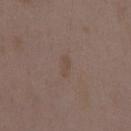workup — no biopsy performed (imaged during a skin exam) | image-analysis metrics — a lesion area of about 3 mm², an outline eccentricity of about 0.9 (0 = round, 1 = elongated), and a shape-asymmetry score of about 0.3 (0 = symmetric); a mean CIELAB color near L≈46 a*≈14 b*≈23, about 4 CIELAB-L* units darker than the surrounding skin, and a lesion-to-skin contrast of about 4.5 (normalized; higher = more distinct); an automated nevus-likeness rating near 0 out of 100 and a lesion-detection confidence of about 100/100 | illumination — white-light | subject — female, approximately 35 years of age | site — the mid back | image source — 15 mm crop, total-body photography | lesion size — ~3 mm (longest diameter).